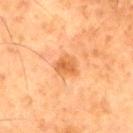<tbp_lesion>
<biopsy_status>not biopsied; imaged during a skin examination</biopsy_status>
<image>
  <source>total-body photography crop</source>
  <field_of_view_mm>15</field_of_view_mm>
</image>
<lesion_size>
  <long_diameter_mm_approx>2.5</long_diameter_mm_approx>
</lesion_size>
<site>right thigh</site>
<lighting>cross-polarized</lighting>
<patient>
  <sex>male</sex>
  <age_approx>80</age_approx>
</patient>
</tbp_lesion>